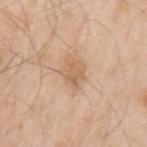Clinical impression:
Recorded during total-body skin imaging; not selected for excision or biopsy.
Acquisition and patient details:
A male patient, aged 53 to 57. Longest diameter approximately 3 mm. Captured under white-light illumination. Located on the left upper arm. The total-body-photography lesion software estimated a lesion–skin lightness drop of about 8. The analysis additionally found an automated nevus-likeness rating near 5 out of 100 and a lesion-detection confidence of about 100/100. Cropped from a whole-body photographic skin survey; the tile spans about 15 mm.